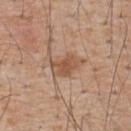Assessment: No biopsy was performed on this lesion — it was imaged during a full skin examination and was not determined to be concerning. Clinical summary: A male subject, aged around 55. The tile uses white-light illumination. About 4 mm across. This image is a 15 mm lesion crop taken from a total-body photograph. On the upper back.